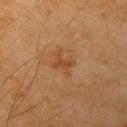Assessment:
The lesion was photographed on a routine skin check and not biopsied; there is no pathology result.
Context:
A male patient aged 68 to 72. Automated image analysis of the tile measured a lesion–skin lightness drop of about 6. The analysis additionally found internal color variation of about 1.5 on a 0–10 scale and peripheral color asymmetry of about 0.5. The analysis additionally found a lesion-detection confidence of about 100/100. The tile uses cross-polarized illumination. Approximately 3 mm at its widest. On the left upper arm. This image is a 15 mm lesion crop taken from a total-body photograph.